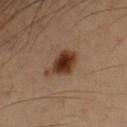Assessment: The lesion was tiled from a total-body skin photograph and was not biopsied. Clinical summary: A 15 mm close-up tile from a total-body photography series done for melanoma screening. A male subject, aged approximately 55. From the left forearm. The lesion-visualizer software estimated an area of roughly 8.5 mm² and an outline eccentricity of about 0.55 (0 = round, 1 = elongated). The software also gave a lesion color around L≈28 a*≈16 b*≈24 in CIELAB, a lesion–skin lightness drop of about 12, and a normalized border contrast of about 11.5. Longest diameter approximately 3.5 mm.